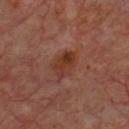<record>
<biopsy_status>not biopsied; imaged during a skin examination</biopsy_status>
</record>